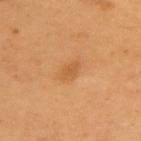This lesion was catalogued during total-body skin photography and was not selected for biopsy.
The lesion-visualizer software estimated a lesion area of about 3 mm², a shape eccentricity near 0.8, and a symmetry-axis asymmetry near 0.35. And it measured a mean CIELAB color near L≈55 a*≈24 b*≈42, a lesion–skin lightness drop of about 7, and a normalized border contrast of about 5.5. The software also gave a nevus-likeness score of about 5/100 and a detector confidence of about 100 out of 100 that the crop contains a lesion.
A male patient roughly 55 years of age.
This is a cross-polarized tile.
The lesion is on the upper back.
This image is a 15 mm lesion crop taken from a total-body photograph.
The lesion's longest dimension is about 2.5 mm.